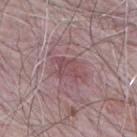Assessment: No biopsy was performed on this lesion — it was imaged during a full skin examination and was not determined to be concerning. Context: Located on the mid back. Longest diameter approximately 4 mm. The lesion-visualizer software estimated a lesion color around L≈48 a*≈22 b*≈15 in CIELAB, about 8 CIELAB-L* units darker than the surrounding skin, and a lesion-to-skin contrast of about 6 (normalized; higher = more distinct). The patient is a male aged approximately 65. A roughly 15 mm field-of-view crop from a total-body skin photograph.